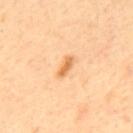The lesion was photographed on a routine skin check and not biopsied; there is no pathology result. A male subject in their 60s. Longest diameter approximately 3 mm. Imaged with cross-polarized lighting. On the mid back. A lesion tile, about 15 mm wide, cut from a 3D total-body photograph.